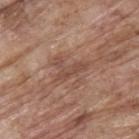{
  "biopsy_status": "not biopsied; imaged during a skin examination",
  "lesion_size": {
    "long_diameter_mm_approx": 4.0
  },
  "lighting": "white-light",
  "site": "upper back",
  "automated_metrics": {
    "area_mm2_approx": 6.0,
    "eccentricity": 0.75,
    "shape_asymmetry": 0.6,
    "cielab_L": 48,
    "cielab_a": 20,
    "cielab_b": 27,
    "vs_skin_darker_L": 8.0,
    "nevus_likeness_0_100": 0
  },
  "patient": {
    "sex": "male",
    "age_approx": 70
  },
  "image": {
    "source": "total-body photography crop",
    "field_of_view_mm": 15
  }
}